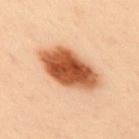workup: total-body-photography surveillance lesion; no biopsy | imaging modality: 15 mm crop, total-body photography | illumination: cross-polarized illumination | lesion diameter: ≈7.5 mm | location: the upper back | patient: male, aged 48 to 52.